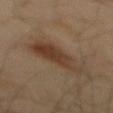Clinical impression: The lesion was photographed on a routine skin check and not biopsied; there is no pathology result. Image and clinical context: The tile uses cross-polarized illumination. The patient is a male approximately 40 years of age. A roughly 15 mm field-of-view crop from a total-body skin photograph. The recorded lesion diameter is about 7.5 mm. The lesion is on the mid back. Automated image analysis of the tile measured a lesion area of about 16 mm², a shape eccentricity near 0.9, and two-axis asymmetry of about 0.3. The software also gave a peripheral color-asymmetry measure near 1.5.